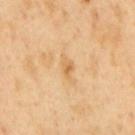biopsy status = catalogued during a skin exam; not biopsied
body site = the mid back
imaging modality = total-body-photography crop, ~15 mm field of view
patient = male, in their 50s